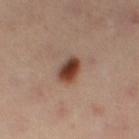Captured during whole-body skin photography for melanoma surveillance; the lesion was not biopsied.
Automated image analysis of the tile measured a mean CIELAB color near L≈40 a*≈21 b*≈28 and about 16 CIELAB-L* units darker than the surrounding skin. The analysis additionally found a border-irregularity index near 1.5/10, a color-variation rating of about 6/10, and a peripheral color-asymmetry measure near 1.5. And it measured a classifier nevus-likeness of about 100/100.
The lesion's longest dimension is about 3.5 mm.
A close-up tile cropped from a whole-body skin photograph, about 15 mm across.
From the right thigh.
A female subject in their 40s.
This is a cross-polarized tile.